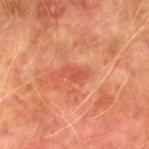| feature | finding |
|---|---|
| notes | catalogued during a skin exam; not biopsied |
| body site | the right lower leg |
| lesion diameter | ≈3 mm |
| patient | male, aged 73 to 77 |
| tile lighting | cross-polarized |
| acquisition | 15 mm crop, total-body photography |